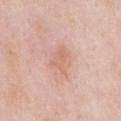<record>
  <biopsy_status>not biopsied; imaged during a skin examination</biopsy_status>
  <image>
    <source>total-body photography crop</source>
    <field_of_view_mm>15</field_of_view_mm>
  </image>
  <patient>
    <sex>male</sex>
    <age_approx>55</age_approx>
  </patient>
  <lighting>white-light</lighting>
  <automated_metrics>
    <cielab_L>66</cielab_L>
    <cielab_a>21</cielab_a>
    <cielab_b>28</cielab_b>
    <vs_skin_darker_L>7.0</vs_skin_darker_L>
    <lesion_detection_confidence_0_100>100</lesion_detection_confidence_0_100>
  </automated_metrics>
  <lesion_size>
    <long_diameter_mm_approx>3.5</long_diameter_mm_approx>
  </lesion_size>
  <site>abdomen</site>
</record>